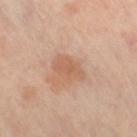workup = imaged on a skin check; not biopsied
body site = the right thigh
subject = female, aged 48–52
lighting = cross-polarized illumination
image source = total-body-photography crop, ~15 mm field of view
size = about 4 mm